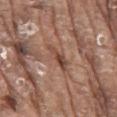Q: Is there a histopathology result?
A: no biopsy performed (imaged during a skin exam)
Q: What kind of image is this?
A: 15 mm crop, total-body photography
Q: Lesion location?
A: the mid back
Q: What did automated image analysis measure?
A: a footprint of about 5.5 mm² and an eccentricity of roughly 0.9
Q: What lighting was used for the tile?
A: white-light illumination
Q: What are the patient's age and sex?
A: male, in their 80s